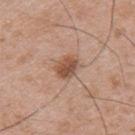follow-up: imaged on a skin check; not biopsied | imaging modality: ~15 mm crop, total-body skin-cancer survey | patient: male, aged 53–57 | anatomic site: the upper back | lighting: white-light.